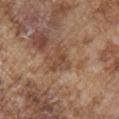biopsy_status: not biopsied; imaged during a skin examination
image:
  source: total-body photography crop
  field_of_view_mm: 15
site: right upper arm
patient:
  sex: male
  age_approx: 75